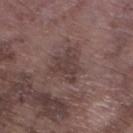biopsy status: total-body-photography surveillance lesion; no biopsy
illumination: white-light illumination
TBP lesion metrics: a footprint of about 8.5 mm², an outline eccentricity of about 0.55 (0 = round, 1 = elongated), and two-axis asymmetry of about 0.5
patient: male, roughly 75 years of age
location: the left lower leg
image: total-body-photography crop, ~15 mm field of view